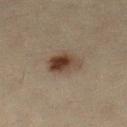Q: Was this lesion biopsied?
A: no biopsy performed (imaged during a skin exam)
Q: How was this image acquired?
A: 15 mm crop, total-body photography
Q: Patient demographics?
A: female, in their mid-60s
Q: How large is the lesion?
A: about 4 mm
Q: Illumination type?
A: cross-polarized
Q: Automated lesion metrics?
A: a lesion area of about 7.5 mm², an eccentricity of roughly 0.8, and two-axis asymmetry of about 0.25
Q: Where on the body is the lesion?
A: the left lower leg